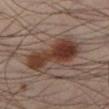Notes:
– workup — total-body-photography surveillance lesion; no biopsy